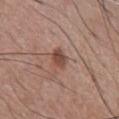| feature | finding |
|---|---|
| follow-up | catalogued during a skin exam; not biopsied |
| subject | male, approximately 65 years of age |
| lighting | white-light |
| TBP lesion metrics | an area of roughly 4 mm² and two-axis asymmetry of about 0.25; a mean CIELAB color near L≈47 a*≈20 b*≈26, about 11 CIELAB-L* units darker than the surrounding skin, and a normalized lesion–skin contrast near 8; an automated nevus-likeness rating near 85 out of 100 and a lesion-detection confidence of about 100/100 |
| anatomic site | the chest |
| imaging modality | ~15 mm tile from a whole-body skin photo |